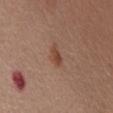Assessment:
Recorded during total-body skin imaging; not selected for excision or biopsy.
Context:
Cropped from a total-body skin-imaging series; the visible field is about 15 mm. The lesion is located on the chest. The subject is a female aged 63 to 67.